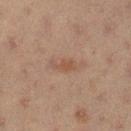Recorded during total-body skin imaging; not selected for excision or biopsy. Imaged with cross-polarized lighting. The subject is a female approximately 20 years of age. Automated tile analysis of the lesion measured a footprint of about 2.5 mm², a shape eccentricity near 0.85, and a symmetry-axis asymmetry near 0.45. And it measured a within-lesion color-variation index near 0.5/10 and a peripheral color-asymmetry measure near 0. On the leg. A lesion tile, about 15 mm wide, cut from a 3D total-body photograph.